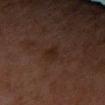Assessment: The lesion was tiled from a total-body skin photograph and was not biopsied. Clinical summary: Cropped from a total-body skin-imaging series; the visible field is about 15 mm. The lesion is located on the right upper arm. A female patient, in their 60s.